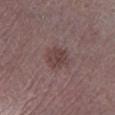Part of a total-body skin-imaging series; this lesion was reviewed on a skin check and was not flagged for biopsy.
A 15 mm crop from a total-body photograph taken for skin-cancer surveillance.
Located on the left lower leg.
About 3 mm across.
A male subject, aged approximately 75.